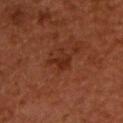workup: total-body-photography surveillance lesion; no biopsy
body site: the upper back
patient: male, roughly 55 years of age
acquisition: total-body-photography crop, ~15 mm field of view
illumination: cross-polarized